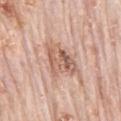workup = imaged on a skin check; not biopsied | acquisition = 15 mm crop, total-body photography | lesion size = about 5 mm | location = the mid back | patient = male, about 80 years old.